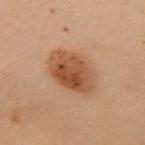biopsy status: no biopsy performed (imaged during a skin exam)
anatomic site: the chest
lesion diameter: ~5.5 mm (longest diameter)
imaging modality: ~15 mm tile from a whole-body skin photo
subject: female, aged around 50
TBP lesion metrics: an area of roughly 18 mm² and an outline eccentricity of about 0.7 (0 = round, 1 = elongated); an average lesion color of about L≈44 a*≈20 b*≈30 (CIELAB) and roughly 10 lightness units darker than nearby skin
illumination: cross-polarized illumination